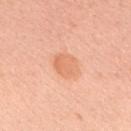The lesion was photographed on a routine skin check and not biopsied; there is no pathology result. A 15 mm close-up tile from a total-body photography series done for melanoma screening. A female patient in their 40s. The lesion is located on the upper back. The total-body-photography lesion software estimated roughly 8 lightness units darker than nearby skin and a normalized border contrast of about 5.5. The software also gave a border-irregularity index near 2/10 and a color-variation rating of about 2.5/10. Measured at roughly 3.5 mm in maximum diameter. This is a white-light tile.